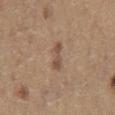Q: Was this lesion biopsied?
A: imaged on a skin check; not biopsied
Q: How was the tile lit?
A: white-light illumination
Q: What is the lesion's diameter?
A: ~3.5 mm (longest diameter)
Q: What did automated image analysis measure?
A: a lesion color around L≈50 a*≈17 b*≈28 in CIELAB, about 9 CIELAB-L* units darker than the surrounding skin, and a normalized border contrast of about 7
Q: Who is the patient?
A: male, about 65 years old
Q: What is the imaging modality?
A: ~15 mm crop, total-body skin-cancer survey
Q: Lesion location?
A: the chest